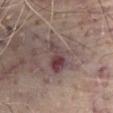Recorded during total-body skin imaging; not selected for excision or biopsy. The subject is a male aged 78 to 82. Approximately 5 mm at its widest. An algorithmic analysis of the crop reported a lesion area of about 10 mm², an eccentricity of roughly 0.85, and two-axis asymmetry of about 0.35. The analysis additionally found an average lesion color of about L≈43 a*≈17 b*≈15 (CIELAB) and roughly 10 lightness units darker than nearby skin. The analysis additionally found an automated nevus-likeness rating near 45 out of 100 and a lesion-detection confidence of about 90/100. Located on the front of the torso. Cropped from a total-body skin-imaging series; the visible field is about 15 mm. Imaged with white-light lighting.